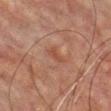The lesion was tiled from a total-body skin photograph and was not biopsied. Approximately 3 mm at its widest. The subject is a male aged 73–77. Captured under cross-polarized illumination. A 15 mm close-up extracted from a 3D total-body photography capture. From the chest.